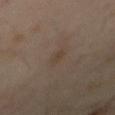Clinical impression: The lesion was tiled from a total-body skin photograph and was not biopsied. Background: Captured under cross-polarized illumination. The total-body-photography lesion software estimated a shape-asymmetry score of about 0.2 (0 = symmetric). It also reported a mean CIELAB color near L≈39 a*≈11 b*≈23 and a lesion-to-skin contrast of about 5 (normalized; higher = more distinct). The analysis additionally found a nevus-likeness score of about 0/100 and a lesion-detection confidence of about 100/100. About 3 mm across. A male patient in their mid-60s. Cropped from a whole-body photographic skin survey; the tile spans about 15 mm.